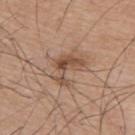workup: imaged on a skin check; not biopsied
lesion size: ≈4 mm
image: total-body-photography crop, ~15 mm field of view
subject: male, about 75 years old
automated lesion analysis: internal color variation of about 6 on a 0–10 scale and a peripheral color-asymmetry measure near 2.5; a nevus-likeness score of about 45/100 and a lesion-detection confidence of about 100/100
site: the upper back